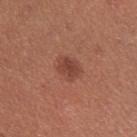- notes · catalogued during a skin exam; not biopsied